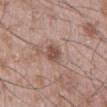{"biopsy_status": "not biopsied; imaged during a skin examination", "patient": {"sex": "male", "age_approx": 50}, "lighting": "white-light", "image": {"source": "total-body photography crop", "field_of_view_mm": 15}, "site": "mid back", "lesion_size": {"long_diameter_mm_approx": 3.0}}